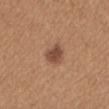Recorded during total-body skin imaging; not selected for excision or biopsy.
A close-up tile cropped from a whole-body skin photograph, about 15 mm across.
Measured at roughly 3 mm in maximum diameter.
On the mid back.
The subject is a female aged 33–37.
This is a white-light tile.